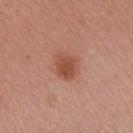Case summary:
- notes — no biopsy performed (imaged during a skin exam)
- image source — ~15 mm crop, total-body skin-cancer survey
- patient — female, aged 38 to 42
- automated metrics — a within-lesion color-variation index near 2.5/10
- illumination — white-light illumination
- lesion size — ~3 mm (longest diameter)
- site — the left forearm